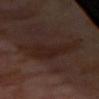Context: An algorithmic analysis of the crop reported a footprint of about 17 mm² and two-axis asymmetry of about 0.45. The analysis additionally found radial color variation of about 0.5. The analysis additionally found a nevus-likeness score of about 5/100. A female patient aged 53 to 57. The lesion's longest dimension is about 8.5 mm. A region of skin cropped from a whole-body photographic capture, roughly 15 mm wide. The lesion is located on the chest.